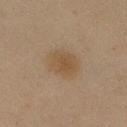Part of a total-body skin-imaging series; this lesion was reviewed on a skin check and was not flagged for biopsy.
The patient is a male roughly 45 years of age.
The total-body-photography lesion software estimated a mean CIELAB color near L≈40 a*≈12 b*≈26, roughly 6 lightness units darker than nearby skin, and a lesion-to-skin contrast of about 6 (normalized; higher = more distinct). The analysis additionally found a nevus-likeness score of about 65/100 and a detector confidence of about 100 out of 100 that the crop contains a lesion.
A 15 mm close-up tile from a total-body photography series done for melanoma screening.
This is a cross-polarized tile.
From the right upper arm.